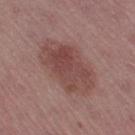{"biopsy_status": "not biopsied; imaged during a skin examination", "lesion_size": {"long_diameter_mm_approx": 7.0}, "automated_metrics": {"cielab_L": 46, "cielab_a": 21, "cielab_b": 22, "vs_skin_darker_L": 9.0, "border_irregularity_0_10": 2.0, "color_variation_0_10": 4.5, "nevus_likeness_0_100": 60, "lesion_detection_confidence_0_100": 100}, "site": "right thigh", "patient": {"sex": "female", "age_approx": 45}, "image": {"source": "total-body photography crop", "field_of_view_mm": 15}, "lighting": "white-light"}